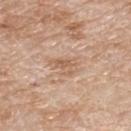Q: Was this lesion biopsied?
A: catalogued during a skin exam; not biopsied